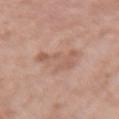No biopsy was performed on this lesion — it was imaged during a full skin examination and was not determined to be concerning.
A female patient aged around 60.
The lesion is located on the arm.
Captured under white-light illumination.
About 5.5 mm across.
This image is a 15 mm lesion crop taken from a total-body photograph.
The lesion-visualizer software estimated an eccentricity of roughly 0.95 and a symmetry-axis asymmetry near 0.5.